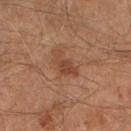Captured during whole-body skin photography for melanoma surveillance; the lesion was not biopsied.
A male subject aged approximately 65.
An algorithmic analysis of the crop reported a border-irregularity rating of about 5/10 and a peripheral color-asymmetry measure near 0.5. And it measured a nevus-likeness score of about 5/100 and lesion-presence confidence of about 100/100.
A 15 mm crop from a total-body photograph taken for skin-cancer surveillance.
Located on the right lower leg.
Longest diameter approximately 3.5 mm.
Captured under cross-polarized illumination.